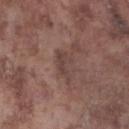Clinical impression: Part of a total-body skin-imaging series; this lesion was reviewed on a skin check and was not flagged for biopsy. Context: The lesion is on the leg. Cropped from a total-body skin-imaging series; the visible field is about 15 mm. The subject is a male aged around 75.